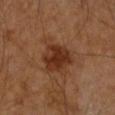Case summary:
– follow-up — total-body-photography surveillance lesion; no biopsy
– subject — male, about 85 years old or older
– acquisition — ~15 mm crop, total-body skin-cancer survey
– anatomic site — the arm